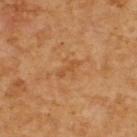Findings:
• biopsy status · no biopsy performed (imaged during a skin exam)
• image source · 15 mm crop, total-body photography
• body site · the upper back
• lesion diameter · about 3 mm
• illumination · cross-polarized
• subject · male, aged around 60